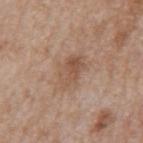Background:
Longest diameter approximately 4.5 mm. The subject is a male in their mid- to late 60s. Cropped from a total-body skin-imaging series; the visible field is about 15 mm. The lesion is on the back. Captured under white-light illumination.